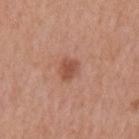Imaged during a routine full-body skin examination; the lesion was not biopsied and no histopathology is available. The lesion's longest dimension is about 2.5 mm. From the back. Captured under white-light illumination. The patient is a male about 70 years old. A 15 mm crop from a total-body photograph taken for skin-cancer surveillance.